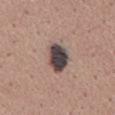{
  "biopsy_status": "not biopsied; imaged during a skin examination"
}